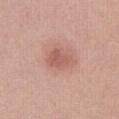Q: Is there a histopathology result?
A: catalogued during a skin exam; not biopsied
Q: What lighting was used for the tile?
A: white-light
Q: How was this image acquired?
A: ~15 mm tile from a whole-body skin photo
Q: Lesion size?
A: about 3.5 mm
Q: Who is the patient?
A: female, roughly 20 years of age
Q: What is the anatomic site?
A: the right thigh